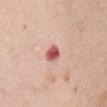Assessment:
The lesion was tiled from a total-body skin photograph and was not biopsied.
Background:
On the chest. Captured under white-light illumination. Longest diameter approximately 2.5 mm. A female patient, in their mid-60s. A 15 mm crop from a total-body photograph taken for skin-cancer surveillance.